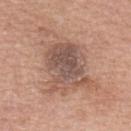Findings:
* follow-up: catalogued during a skin exam; not biopsied
* image: ~15 mm tile from a whole-body skin photo
* lighting: white-light
* TBP lesion metrics: an average lesion color of about L≈55 a*≈19 b*≈26 (CIELAB) and roughly 10 lightness units darker than nearby skin; an automated nevus-likeness rating near 10 out of 100 and a lesion-detection confidence of about 100/100
* lesion size: ≈9 mm
* patient: female, aged around 60
* anatomic site: the left forearm